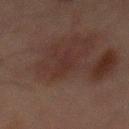Q: Is there a histopathology result?
A: imaged on a skin check; not biopsied
Q: Who is the patient?
A: male, in their mid- to late 60s
Q: What kind of image is this?
A: ~15 mm tile from a whole-body skin photo
Q: What lighting was used for the tile?
A: cross-polarized illumination
Q: Lesion location?
A: the abdomen
Q: What is the lesion's diameter?
A: ~4 mm (longest diameter)